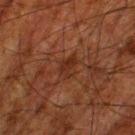Q: Lesion size?
A: ~3 mm (longest diameter)
Q: Who is the patient?
A: male, roughly 80 years of age
Q: What is the imaging modality?
A: ~15 mm tile from a whole-body skin photo
Q: Where on the body is the lesion?
A: the left thigh